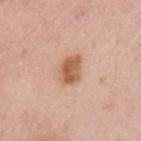{"biopsy_status": "not biopsied; imaged during a skin examination", "site": "left upper arm", "patient": {"sex": "female", "age_approx": 40}, "image": {"source": "total-body photography crop", "field_of_view_mm": 15}}